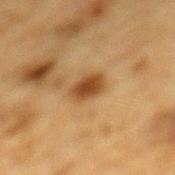Q: Was this lesion biopsied?
A: no biopsy performed (imaged during a skin exam)
Q: Where on the body is the lesion?
A: the mid back
Q: Patient demographics?
A: male, about 85 years old or older
Q: What did automated image analysis measure?
A: an area of roughly 6 mm², a shape eccentricity near 0.7, and a shape-asymmetry score of about 0.2 (0 = symmetric); a mean CIELAB color near L≈41 a*≈20 b*≈35, about 12 CIELAB-L* units darker than the surrounding skin, and a lesion-to-skin contrast of about 10 (normalized; higher = more distinct)
Q: What lighting was used for the tile?
A: cross-polarized illumination
Q: What is the imaging modality?
A: ~15 mm tile from a whole-body skin photo
Q: Lesion size?
A: ≈3 mm